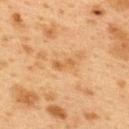workup = total-body-photography surveillance lesion; no biopsy | acquisition = 15 mm crop, total-body photography | patient = female, aged around 40 | body site = the upper back.